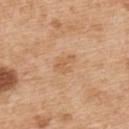workup: total-body-photography surveillance lesion; no biopsy | patient: male, in their mid-50s | TBP lesion metrics: a shape eccentricity near 0.75 and a shape-asymmetry score of about 0.35 (0 = symmetric); about 6 CIELAB-L* units darker than the surrounding skin; a border-irregularity index near 3/10 and peripheral color asymmetry of about 1; an automated nevus-likeness rating near 0 out of 100 and a lesion-detection confidence of about 100/100 | location: the upper back | lesion diameter: ≈3 mm | imaging modality: ~15 mm tile from a whole-body skin photo.